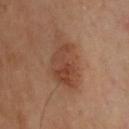patient:
  sex: male
  age_approx: 45
image:
  source: total-body photography crop
  field_of_view_mm: 15
site: upper back